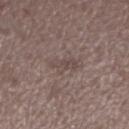Imaged during a routine full-body skin examination; the lesion was not biopsied and no histopathology is available.